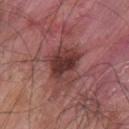workup = no biopsy performed (imaged during a skin exam)
subject = male, aged approximately 45
image-analysis metrics = a border-irregularity rating of about 3.5/10, a color-variation rating of about 6/10, and peripheral color asymmetry of about 2; an automated nevus-likeness rating near 0 out of 100 and lesion-presence confidence of about 100/100
acquisition = 15 mm crop, total-body photography
site = the upper back
diameter = ≈4.5 mm
tile lighting = white-light illumination